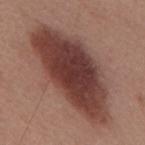lighting: white-light
site: back
lesion_size:
  long_diameter_mm_approx: 12.5
image:
  source: total-body photography crop
  field_of_view_mm: 15
automated_metrics:
  area_mm2_approx: 50.0
  shape_asymmetry: 0.2
  nevus_likeness_0_100: 80
  lesion_detection_confidence_0_100: 100
patient:
  sex: male
  age_approx: 70